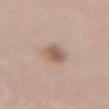<case>
<automated_metrics>
  <area_mm2_approx>5.5</area_mm2_approx>
  <eccentricity>0.85</eccentricity>
  <shape_asymmetry>0.2</shape_asymmetry>
  <nevus_likeness_0_100>100</nevus_likeness_0_100>
</automated_metrics>
<site>lower back</site>
<patient>
  <sex>female</sex>
  <age_approx>40</age_approx>
</patient>
<lesion_size>
  <long_diameter_mm_approx>3.5</long_diameter_mm_approx>
</lesion_size>
<image>
  <source>total-body photography crop</source>
  <field_of_view_mm>15</field_of_view_mm>
</image>
</case>